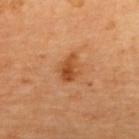biopsy status: no biopsy performed (imaged during a skin exam)
image source: total-body-photography crop, ~15 mm field of view
diameter: ~3 mm (longest diameter)
tile lighting: cross-polarized
subject: aged 68–72
site: the upper back